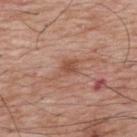notes = catalogued during a skin exam; not biopsied | imaging modality = ~15 mm crop, total-body skin-cancer survey | patient = male, aged around 70 | body site = the upper back.